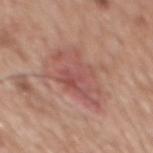The lesion was tiled from a total-body skin photograph and was not biopsied.
The tile uses white-light illumination.
Cropped from a whole-body photographic skin survey; the tile spans about 15 mm.
A male subject aged 58 to 62.
Located on the mid back.